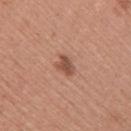Located on the arm. This is a white-light tile. The lesion's longest dimension is about 3 mm. A female patient, about 40 years old. A lesion tile, about 15 mm wide, cut from a 3D total-body photograph.